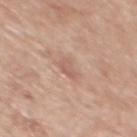Findings:
- workup — no biopsy performed (imaged during a skin exam)
- lighting — white-light
- automated metrics — an area of roughly 3.5 mm², an eccentricity of roughly 0.8, and a shape-asymmetry score of about 0.35 (0 = symmetric); a lesion color around L≈59 a*≈21 b*≈26 in CIELAB, roughly 7 lightness units darker than nearby skin, and a lesion-to-skin contrast of about 5 (normalized; higher = more distinct); an automated nevus-likeness rating near 0 out of 100 and lesion-presence confidence of about 100/100
- anatomic site — the back
- patient — male, roughly 60 years of age
- acquisition — ~15 mm crop, total-body skin-cancer survey
- diameter — about 2.5 mm